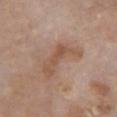No biopsy was performed on this lesion — it was imaged during a full skin examination and was not determined to be concerning. About 5.5 mm across. A female subject, aged approximately 65. A roughly 15 mm field-of-view crop from a total-body skin photograph. The lesion is on the chest.